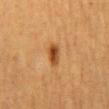biopsy status: catalogued during a skin exam; not biopsied | patient: female, in their mid-50s | site: the mid back | acquisition: 15 mm crop, total-body photography.